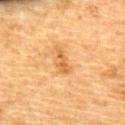Impression:
The lesion was tiled from a total-body skin photograph and was not biopsied.
Acquisition and patient details:
Located on the upper back. This image is a 15 mm lesion crop taken from a total-body photograph. A female subject aged approximately 55.